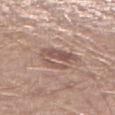Context: Approximately 4 mm at its widest. A male subject aged around 30. Automated tile analysis of the lesion measured a footprint of about 9 mm² and an eccentricity of roughly 0.65. And it measured an average lesion color of about L≈53 a*≈19 b*≈23 (CIELAB), roughly 10 lightness units darker than nearby skin, and a normalized lesion–skin contrast near 7. Located on the right lower leg. A close-up tile cropped from a whole-body skin photograph, about 15 mm across. The tile uses white-light illumination.